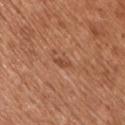| feature | finding |
|---|---|
| image source | total-body-photography crop, ~15 mm field of view |
| patient | male, roughly 55 years of age |
| body site | the chest |